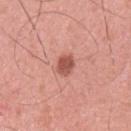Notes:
• workup — total-body-photography surveillance lesion; no biopsy
• patient — male, about 35 years old
• image-analysis metrics — a lesion area of about 4.5 mm², an eccentricity of roughly 0.7, and two-axis asymmetry of about 0.2; a lesion–skin lightness drop of about 13 and a normalized border contrast of about 8.5; a classifier nevus-likeness of about 95/100 and lesion-presence confidence of about 100/100
• body site — the arm
• image source — 15 mm crop, total-body photography